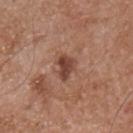Impression: Captured during whole-body skin photography for melanoma surveillance; the lesion was not biopsied. Background: This image is a 15 mm lesion crop taken from a total-body photograph. Located on the chest. Captured under white-light illumination. A male subject, about 55 years old.